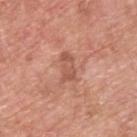The lesion was photographed on a routine skin check and not biopsied; there is no pathology result. A male patient in their mid-50s. The lesion is on the upper back. This image is a 15 mm lesion crop taken from a total-body photograph. Longest diameter approximately 3.5 mm. Captured under white-light illumination.